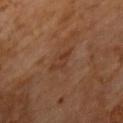Findings:
– biopsy status — imaged on a skin check; not biopsied
– imaging modality — ~15 mm crop, total-body skin-cancer survey
– anatomic site — the back
– patient — male, aged 58 to 62
– size — ~3.5 mm (longest diameter)